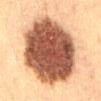Notes:
– notes: total-body-photography surveillance lesion; no biopsy
– tile lighting: cross-polarized
– patient: female, in their 30s
– acquisition: total-body-photography crop, ~15 mm field of view
– diameter: ~10 mm (longest diameter)
– automated lesion analysis: a shape eccentricity near 0.5 and a symmetry-axis asymmetry near 0.1; a lesion-detection confidence of about 100/100
– site: the abdomen